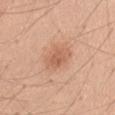notes: imaged on a skin check; not biopsied
size: about 3.5 mm
subject: male, aged 48 to 52
image-analysis metrics: a classifier nevus-likeness of about 55/100 and lesion-presence confidence of about 100/100
image source: ~15 mm crop, total-body skin-cancer survey
tile lighting: white-light illumination
body site: the abdomen